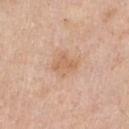Assessment:
This lesion was catalogued during total-body skin photography and was not selected for biopsy.